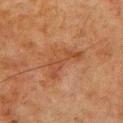biopsy status = imaged on a skin check; not biopsied | image source = 15 mm crop, total-body photography | illumination = cross-polarized | TBP lesion metrics = an average lesion color of about L≈38 a*≈21 b*≈30 (CIELAB), roughly 6 lightness units darker than nearby skin, and a normalized lesion–skin contrast near 5.5; border irregularity of about 7 on a 0–10 scale and peripheral color asymmetry of about 0.5 | subject = male, in their 80s | location = the chest.